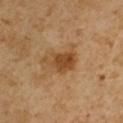{
  "biopsy_status": "not biopsied; imaged during a skin examination",
  "lesion_size": {
    "long_diameter_mm_approx": 4.0
  },
  "automated_metrics": {
    "area_mm2_approx": 8.0,
    "color_variation_0_10": 5.0,
    "peripheral_color_asymmetry": 2.0,
    "nevus_likeness_0_100": 55,
    "lesion_detection_confidence_0_100": 100
  },
  "lighting": "cross-polarized",
  "site": "arm",
  "patient": {
    "sex": "male",
    "age_approx": 50
  },
  "image": {
    "source": "total-body photography crop",
    "field_of_view_mm": 15
  }
}